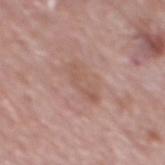This lesion was catalogued during total-body skin photography and was not selected for biopsy. A male subject approximately 70 years of age. Cropped from a whole-body photographic skin survey; the tile spans about 15 mm. The lesion is located on the mid back.